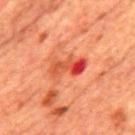notes: no biopsy performed (imaged during a skin exam) | subject: male, aged approximately 65 | size: about 4.5 mm | acquisition: ~15 mm crop, total-body skin-cancer survey | tile lighting: cross-polarized illumination | anatomic site: the mid back.